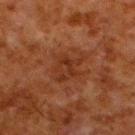A 15 mm crop from a total-body photograph taken for skin-cancer surveillance.
The lesion is located on the left lower leg.
A male patient aged around 80.